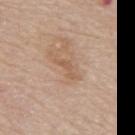workup: imaged on a skin check; not biopsied | acquisition: ~15 mm crop, total-body skin-cancer survey | patient: male, aged 73 to 77 | anatomic site: the mid back.